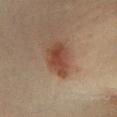biopsy status: total-body-photography surveillance lesion; no biopsy
site: the left lower leg
imaging modality: ~15 mm tile from a whole-body skin photo
automated lesion analysis: an area of roughly 10 mm² and two-axis asymmetry of about 0.25; a nevus-likeness score of about 100/100
diameter: ~4.5 mm (longest diameter)
subject: female, aged around 40